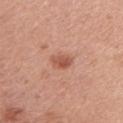Assessment: Imaged during a routine full-body skin examination; the lesion was not biopsied and no histopathology is available. Acquisition and patient details: Longest diameter approximately 2.5 mm. Cropped from a whole-body photographic skin survey; the tile spans about 15 mm. The subject is a female about 60 years old. The lesion is on the arm. Captured under white-light illumination.